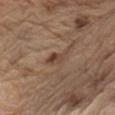follow-up = catalogued during a skin exam; not biopsied | body site = the left upper arm | lighting = white-light illumination | patient = male, approximately 70 years of age | imaging modality = total-body-photography crop, ~15 mm field of view | lesion diameter = ~4 mm (longest diameter).